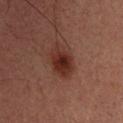Findings:
– workup: no biopsy performed (imaged during a skin exam)
– diameter: ≈4 mm
– tile lighting: cross-polarized
– location: the right upper arm
– image source: total-body-photography crop, ~15 mm field of view
– subject: male, aged 33–37